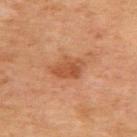Captured during whole-body skin photography for melanoma surveillance; the lesion was not biopsied. On the upper back. The patient is a female approximately 60 years of age. A lesion tile, about 15 mm wide, cut from a 3D total-body photograph. The recorded lesion diameter is about 4 mm. Automated tile analysis of the lesion measured a lesion area of about 7.5 mm², a shape eccentricity near 0.8, and a shape-asymmetry score of about 0.3 (0 = symmetric).